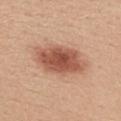biopsy status: total-body-photography surveillance lesion; no biopsy | patient: female, in their 30s | tile lighting: white-light | body site: the upper back | image source: ~15 mm crop, total-body skin-cancer survey | diameter: ≈7 mm.